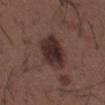biopsy status = no biopsy performed (imaged during a skin exam); body site = the mid back; lighting = white-light; subject = male, roughly 50 years of age; size = ~5.5 mm (longest diameter); image source = total-body-photography crop, ~15 mm field of view.